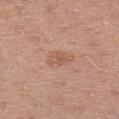The lesion was photographed on a routine skin check and not biopsied; there is no pathology result.
The subject is a male approximately 30 years of age.
This is a white-light tile.
A close-up tile cropped from a whole-body skin photograph, about 15 mm across.
From the left upper arm.
About 3 mm across.